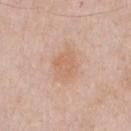Imaged during a routine full-body skin examination; the lesion was not biopsied and no histopathology is available.
An algorithmic analysis of the crop reported border irregularity of about 2 on a 0–10 scale, internal color variation of about 2 on a 0–10 scale, and radial color variation of about 0.5.
Cropped from a whole-body photographic skin survey; the tile spans about 15 mm.
The lesion is located on the front of the torso.
This is a white-light tile.
A male patient, aged approximately 60.
Approximately 4.5 mm at its widest.